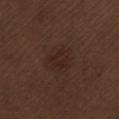workup = total-body-photography surveillance lesion; no biopsy | image = ~15 mm crop, total-body skin-cancer survey | patient = male, about 70 years old | lesion size = ≈2.5 mm | lighting = white-light | site = the lower back.